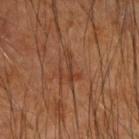<record>
  <biopsy_status>not biopsied; imaged during a skin examination</biopsy_status>
  <patient>
    <sex>male</sex>
    <age_approx>60</age_approx>
  </patient>
  <image>
    <source>total-body photography crop</source>
    <field_of_view_mm>15</field_of_view_mm>
  </image>
  <site>right forearm</site>
</record>